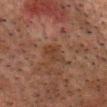Assessment: The lesion was photographed on a routine skin check and not biopsied; there is no pathology result. Image and clinical context: A male subject, aged 58–62. Automated image analysis of the tile measured an automated nevus-likeness rating near 0 out of 100 and lesion-presence confidence of about 100/100. Cropped from a whole-body photographic skin survey; the tile spans about 15 mm. The tile uses cross-polarized illumination. On the head or neck.